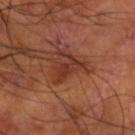{
  "patient": {
    "sex": "male",
    "age_approx": 55
  },
  "image": {
    "source": "total-body photography crop",
    "field_of_view_mm": 15
  },
  "site": "arm",
  "automated_metrics": {
    "cielab_L": 32,
    "cielab_a": 23,
    "cielab_b": 27
  },
  "lesion_size": {
    "long_diameter_mm_approx": 4.5
  }
}